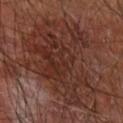<case>
  <biopsy_status>not biopsied; imaged during a skin examination</biopsy_status>
  <image>
    <source>total-body photography crop</source>
    <field_of_view_mm>15</field_of_view_mm>
  </image>
  <lighting>cross-polarized</lighting>
  <site>right forearm</site>
  <patient>
    <sex>male</sex>
    <age_approx>65</age_approx>
  </patient>
</case>